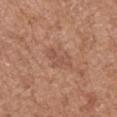The lesion was photographed on a routine skin check and not biopsied; there is no pathology result.
The total-body-photography lesion software estimated an average lesion color of about L≈52 a*≈22 b*≈29 (CIELAB), about 7 CIELAB-L* units darker than the surrounding skin, and a normalized lesion–skin contrast near 5. The analysis additionally found a classifier nevus-likeness of about 0/100 and a detector confidence of about 100 out of 100 that the crop contains a lesion.
The lesion is on the left upper arm.
A male subject, aged 73–77.
Approximately 3.5 mm at its widest.
Captured under white-light illumination.
Cropped from a whole-body photographic skin survey; the tile spans about 15 mm.